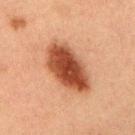Case summary:
* notes · imaged on a skin check; not biopsied
* lesion size · ≈7 mm
* subject · male, roughly 50 years of age
* image source · 15 mm crop, total-body photography
* illumination · cross-polarized
* anatomic site · the upper back
* automated metrics · a mean CIELAB color near L≈38 a*≈23 b*≈29, roughly 15 lightness units darker than nearby skin, and a normalized border contrast of about 12